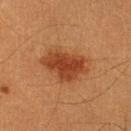Findings:
– workup — total-body-photography surveillance lesion; no biopsy
– TBP lesion metrics — a normalized lesion–skin contrast near 8.5; lesion-presence confidence of about 100/100
– tile lighting — cross-polarized
– diameter — ~6 mm (longest diameter)
– patient — male, aged 38–42
– image source — total-body-photography crop, ~15 mm field of view
– anatomic site — the right lower leg